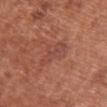No biopsy was performed on this lesion — it was imaged during a full skin examination and was not determined to be concerning.
The subject is a female aged around 65.
Cropped from a whole-body photographic skin survey; the tile spans about 15 mm.
The lesion is located on the chest.